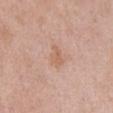Impression: Imaged during a routine full-body skin examination; the lesion was not biopsied and no histopathology is available. Context: Cropped from a total-body skin-imaging series; the visible field is about 15 mm. The lesion is located on the chest. A female subject, aged approximately 65.